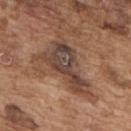follow-up = no biopsy performed (imaged during a skin exam) | subject = male, roughly 75 years of age | acquisition = total-body-photography crop, ~15 mm field of view | site = the upper back | tile lighting = white-light illumination.